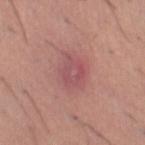Recorded during total-body skin imaging; not selected for excision or biopsy. A male patient, aged approximately 45. The lesion is located on the right thigh. The lesion's longest dimension is about 4.5 mm. A close-up tile cropped from a whole-body skin photograph, about 15 mm across. Automated tile analysis of the lesion measured internal color variation of about 4 on a 0–10 scale and peripheral color asymmetry of about 1.5. The software also gave an automated nevus-likeness rating near 0 out of 100 and a detector confidence of about 100 out of 100 that the crop contains a lesion.